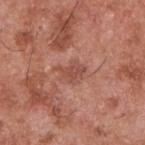A male patient, aged approximately 55. Located on the upper back. Approximately 3.5 mm at its widest. Cropped from a whole-body photographic skin survey; the tile spans about 15 mm.